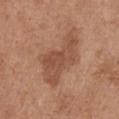Clinical impression: This lesion was catalogued during total-body skin photography and was not selected for biopsy. Image and clinical context: The lesion is located on the chest. A female patient aged approximately 65. A 15 mm crop from a total-body photograph taken for skin-cancer surveillance.